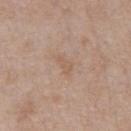{"biopsy_status": "not biopsied; imaged during a skin examination", "lesion_size": {"long_diameter_mm_approx": 2.5}, "image": {"source": "total-body photography crop", "field_of_view_mm": 15}, "patient": {"sex": "male", "age_approx": 65}, "site": "chest"}